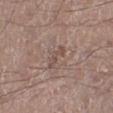workup: imaged on a skin check; not biopsied | site: the left lower leg | image-analysis metrics: a shape eccentricity near 0.9 and two-axis asymmetry of about 0.5; an average lesion color of about L≈49 a*≈16 b*≈23 (CIELAB), about 7 CIELAB-L* units darker than the surrounding skin, and a normalized border contrast of about 5.5; an automated nevus-likeness rating near 0 out of 100 and lesion-presence confidence of about 95/100 | subject: male, approximately 65 years of age | lighting: white-light illumination | imaging modality: ~15 mm tile from a whole-body skin photo | lesion diameter: ≈3.5 mm.